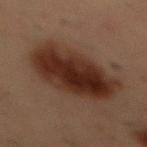workup = total-body-photography surveillance lesion; no biopsy
anatomic site = the front of the torso
lighting = cross-polarized
imaging modality = ~15 mm tile from a whole-body skin photo
lesion size = ~8 mm (longest diameter)
patient = male, approximately 55 years of age